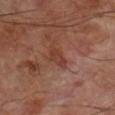Assessment: The lesion was tiled from a total-body skin photograph and was not biopsied. Background: Longest diameter approximately 3 mm. A male patient approximately 70 years of age. Cropped from a whole-body photographic skin survey; the tile spans about 15 mm. From the right lower leg.